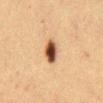The lesion was photographed on a routine skin check and not biopsied; there is no pathology result. Captured under cross-polarized illumination. From the abdomen. About 3.5 mm across. A female subject approximately 40 years of age. A 15 mm close-up extracted from a 3D total-body photography capture.